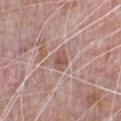workup — imaged on a skin check; not biopsied
location — the chest
subject — male, roughly 70 years of age
image — 15 mm crop, total-body photography